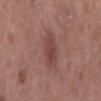Q: Is there a histopathology result?
A: total-body-photography surveillance lesion; no biopsy
Q: What is the anatomic site?
A: the mid back
Q: What is the imaging modality?
A: total-body-photography crop, ~15 mm field of view
Q: Who is the patient?
A: male, aged 58 to 62